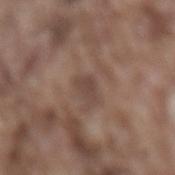Q: Is there a histopathology result?
A: imaged on a skin check; not biopsied
Q: What is the anatomic site?
A: the lower back
Q: Automated lesion metrics?
A: a border-irregularity rating of about 4/10 and peripheral color asymmetry of about 0.5; an automated nevus-likeness rating near 0 out of 100 and lesion-presence confidence of about 100/100
Q: Who is the patient?
A: male, in their mid-70s
Q: How large is the lesion?
A: ~2.5 mm (longest diameter)
Q: What is the imaging modality?
A: total-body-photography crop, ~15 mm field of view
Q: How was the tile lit?
A: white-light illumination